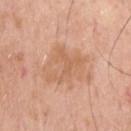Imaged during a routine full-body skin examination; the lesion was not biopsied and no histopathology is available. The lesion-visualizer software estimated a border-irregularity rating of about 9/10, a within-lesion color-variation index near 1.5/10, and radial color variation of about 0.5. The tile uses white-light illumination. From the upper back. The recorded lesion diameter is about 4 mm. A 15 mm crop from a total-body photograph taken for skin-cancer surveillance. The subject is a male aged 68 to 72.